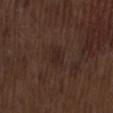Part of a total-body skin-imaging series; this lesion was reviewed on a skin check and was not flagged for biopsy.
The lesion-visualizer software estimated a lesion area of about 4.5 mm², an outline eccentricity of about 0.6 (0 = round, 1 = elongated), and a symmetry-axis asymmetry near 0.25. And it measured an average lesion color of about L≈24 a*≈15 b*≈19 (CIELAB). And it measured a within-lesion color-variation index near 3/10 and radial color variation of about 1. The analysis additionally found an automated nevus-likeness rating near 5 out of 100.
Longest diameter approximately 2.5 mm.
The lesion is on the mid back.
A 15 mm close-up extracted from a 3D total-body photography capture.
A male subject in their 70s.
The tile uses white-light illumination.